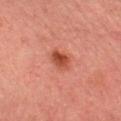Q: Was a biopsy performed?
A: catalogued during a skin exam; not biopsied